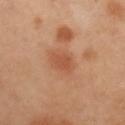  image:
    source: total-body photography crop
    field_of_view_mm: 15
  lighting: cross-polarized
  lesion_size:
    long_diameter_mm_approx: 3.0
  automated_metrics:
    vs_skin_darker_L: 6.0
    vs_skin_contrast_norm: 5.5
    nevus_likeness_0_100: 5
    lesion_detection_confidence_0_100: 100
  patient:
    sex: male
    age_approx: 55
  site: right upper arm